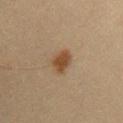Assessment: The lesion was tiled from a total-body skin photograph and was not biopsied. Context: A close-up tile cropped from a whole-body skin photograph, about 15 mm across. On the chest. Approximately 3.5 mm at its widest. A male subject in their mid-30s. This is a cross-polarized tile.